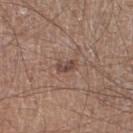The lesion is located on the right lower leg. Cropped from a whole-body photographic skin survey; the tile spans about 15 mm. A male subject about 65 years old.